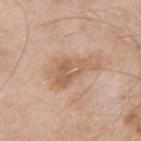This lesion was catalogued during total-body skin photography and was not selected for biopsy. The subject is a male aged approximately 55. Measured at roughly 5.5 mm in maximum diameter. The lesion-visualizer software estimated a border-irregularity rating of about 5.5/10 and a within-lesion color-variation index near 4.5/10. And it measured a nevus-likeness score of about 0/100. Cropped from a whole-body photographic skin survey; the tile spans about 15 mm. Captured under white-light illumination. The lesion is on the left upper arm.